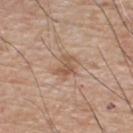notes: total-body-photography surveillance lesion; no biopsy.